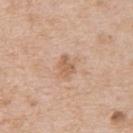workup=no biopsy performed (imaged during a skin exam) | subject=male, about 65 years old | image source=~15 mm tile from a whole-body skin photo | body site=the upper back.